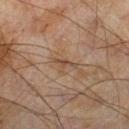Clinical impression:
Captured during whole-body skin photography for melanoma surveillance; the lesion was not biopsied.
Image and clinical context:
A lesion tile, about 15 mm wide, cut from a 3D total-body photograph. From the leg. Captured under cross-polarized illumination. The subject is a male approximately 45 years of age.